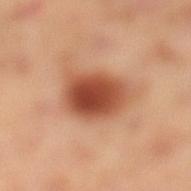  site: leg
  patient:
    sex: female
    age_approx: 40
  image:
    source: total-body photography crop
    field_of_view_mm: 15
  lighting: cross-polarized
  lesion_size:
    long_diameter_mm_approx: 5.5
  automated_metrics:
    area_mm2_approx: 19.0
    eccentricity: 0.55
    cielab_L: 50
    cielab_a: 27
    cielab_b: 34
    vs_skin_darker_L: 15.0
    vs_skin_contrast_norm: 10.5
    border_irregularity_0_10: 2.0
    peripheral_color_asymmetry: 1.5
  diagnosis:
    histopathology: compound melanocytic nevus
    malignancy: benign
    taxonomic_path:
      - Benign
      - Benign melanocytic proliferations
      - Nevus
      - Nevus, NOS, Compound